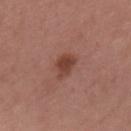Clinical impression:
Imaged during a routine full-body skin examination; the lesion was not biopsied and no histopathology is available.
Clinical summary:
Longest diameter approximately 3.5 mm. The lesion is located on the upper back. Imaged with white-light lighting. A female subject approximately 40 years of age. A 15 mm close-up tile from a total-body photography series done for melanoma screening. The total-body-photography lesion software estimated an outline eccentricity of about 0.75 (0 = round, 1 = elongated) and a shape-asymmetry score of about 0.35 (0 = symmetric). The analysis additionally found a lesion–skin lightness drop of about 10.